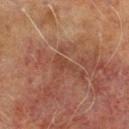Captured during whole-body skin photography for melanoma surveillance; the lesion was not biopsied.
An algorithmic analysis of the crop reported a footprint of about 3 mm² and an outline eccentricity of about 0.9 (0 = round, 1 = elongated). And it measured internal color variation of about 1 on a 0–10 scale and a peripheral color-asymmetry measure near 0.5.
A male patient aged 68–72.
This is a cross-polarized tile.
Longest diameter approximately 3 mm.
A 15 mm close-up tile from a total-body photography series done for melanoma screening.
The lesion is located on the left lower leg.